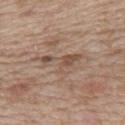* notes: imaged on a skin check; not biopsied
* imaging modality: total-body-photography crop, ~15 mm field of view
* automated lesion analysis: a border-irregularity rating of about 7/10, a within-lesion color-variation index near 7/10, and radial color variation of about 2.5
* subject: female, aged approximately 40
* anatomic site: the back